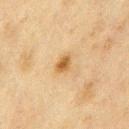body site — the chest
patient — male, about 75 years old
image — 15 mm crop, total-body photography
lesion size — about 2.5 mm
illumination — cross-polarized